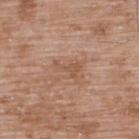<case>
  <biopsy_status>not biopsied; imaged during a skin examination</biopsy_status>
  <automated_metrics>
    <cielab_L>53</cielab_L>
    <cielab_a>21</cielab_a>
    <cielab_b>31</cielab_b>
    <vs_skin_darker_L>7.0</vs_skin_darker_L>
    <vs_skin_contrast_norm>5.5</vs_skin_contrast_norm>
    <border_irregularity_0_10>7.5</border_irregularity_0_10>
    <color_variation_0_10>0.5</color_variation_0_10>
    <peripheral_color_asymmetry>0.0</peripheral_color_asymmetry>
    <nevus_likeness_0_100>0</nevus_likeness_0_100>
  </automated_metrics>
  <patient>
    <sex>male</sex>
    <age_approx>50</age_approx>
  </patient>
  <site>upper back</site>
  <image>
    <source>total-body photography crop</source>
    <field_of_view_mm>15</field_of_view_mm>
  </image>
  <lighting>white-light</lighting>
</case>